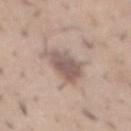Image and clinical context: A male patient approximately 30 years of age. On the abdomen. About 4 mm across. A 15 mm close-up tile from a total-body photography series done for melanoma screening. The total-body-photography lesion software estimated a lesion area of about 11 mm² and a shape-asymmetry score of about 0.3 (0 = symmetric). The analysis additionally found a mean CIELAB color near L≈56 a*≈16 b*≈22, a lesion–skin lightness drop of about 12, and a normalized border contrast of about 8. The analysis additionally found border irregularity of about 3 on a 0–10 scale, a color-variation rating of about 3.5/10, and radial color variation of about 1. And it measured lesion-presence confidence of about 100/100.